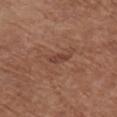Notes:
* notes · imaged on a skin check; not biopsied
* lighting · white-light
* imaging modality · total-body-photography crop, ~15 mm field of view
* size · about 2.5 mm
* site · the chest
* subject · female, in their mid-70s